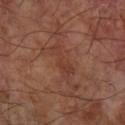Findings:
– workup — imaged on a skin check; not biopsied
– anatomic site — the right forearm
– imaging modality — ~15 mm tile from a whole-body skin photo
– TBP lesion metrics — a footprint of about 6 mm² and a shape eccentricity near 0.95; an average lesion color of about L≈36 a*≈22 b*≈26 (CIELAB), about 5 CIELAB-L* units darker than the surrounding skin, and a lesion-to-skin contrast of about 5 (normalized; higher = more distinct); border irregularity of about 5.5 on a 0–10 scale, a color-variation rating of about 1/10, and a peripheral color-asymmetry measure near 0
– diameter — ~4.5 mm (longest diameter)
– illumination — cross-polarized illumination
– subject — male, aged 63–67